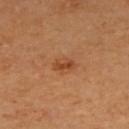Imaged during a routine full-body skin examination; the lesion was not biopsied and no histopathology is available. Located on the right upper arm. Imaged with cross-polarized lighting. A male patient, roughly 65 years of age. About 2.5 mm across. Cropped from a total-body skin-imaging series; the visible field is about 15 mm. The total-body-photography lesion software estimated an area of roughly 3.5 mm². The analysis additionally found a mean CIELAB color near L≈46 a*≈25 b*≈38, about 9 CIELAB-L* units darker than the surrounding skin, and a normalized lesion–skin contrast near 6.5. And it measured a border-irregularity index near 2/10, a within-lesion color-variation index near 3/10, and radial color variation of about 1. And it measured an automated nevus-likeness rating near 80 out of 100 and lesion-presence confidence of about 100/100.